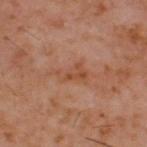The lesion was tiled from a total-body skin photograph and was not biopsied.
A 15 mm close-up tile from a total-body photography series done for melanoma screening.
The lesion is located on the upper back.
Imaged with cross-polarized lighting.
About 4.5 mm across.
A male subject aged around 60.